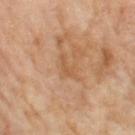The lesion was tiled from a total-body skin photograph and was not biopsied. A 15 mm crop from a total-body photograph taken for skin-cancer surveillance. The lesion's longest dimension is about 3 mm. Automated tile analysis of the lesion measured a lesion color around L≈57 a*≈21 b*≈37 in CIELAB and a lesion–skin lightness drop of about 7. And it measured a detector confidence of about 95 out of 100 that the crop contains a lesion. The subject is a female aged approximately 70. The lesion is on the left thigh. This is a cross-polarized tile.